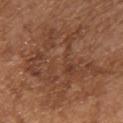biopsy_status: not biopsied; imaged during a skin examination
site: front of the torso
automated_metrics:
  cielab_L: 42
  cielab_a: 21
  cielab_b: 30
  vs_skin_darker_L: 7.0
  vs_skin_contrast_norm: 6.0
lesion_size:
  long_diameter_mm_approx: 11.0
patient:
  sex: male
  age_approx: 70
lighting: white-light
image:
  source: total-body photography crop
  field_of_view_mm: 15